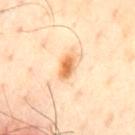Notes:
• workup · imaged on a skin check; not biopsied
• tile lighting · cross-polarized
• subject · male, aged approximately 65
• location · the front of the torso
• imaging modality · ~15 mm tile from a whole-body skin photo
• lesion size · ≈3 mm